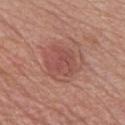Assessment: Captured during whole-body skin photography for melanoma surveillance; the lesion was not biopsied. Clinical summary: A 15 mm close-up tile from a total-body photography series done for melanoma screening. The lesion's longest dimension is about 3.5 mm. This is a white-light tile. From the front of the torso. A male subject, in their mid-70s.